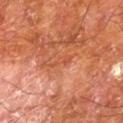No biopsy was performed on this lesion — it was imaged during a full skin examination and was not determined to be concerning.